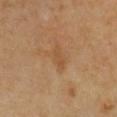No biopsy was performed on this lesion — it was imaged during a full skin examination and was not determined to be concerning. A region of skin cropped from a whole-body photographic capture, roughly 15 mm wide. The subject is a female approximately 50 years of age. Measured at roughly 2.5 mm in maximum diameter. On the chest. Imaged with cross-polarized lighting.